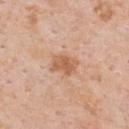{"biopsy_status": "not biopsied; imaged during a skin examination", "lighting": "white-light", "patient": {"sex": "male", "age_approx": 60}, "lesion_size": {"long_diameter_mm_approx": 3.5}, "automated_metrics": {"eccentricity": 0.75, "shape_asymmetry": 0.35, "border_irregularity_0_10": 3.0, "color_variation_0_10": 2.5}, "image": {"source": "total-body photography crop", "field_of_view_mm": 15}, "site": "upper back"}